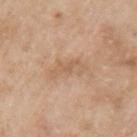Findings:
• follow-up: no biopsy performed (imaged during a skin exam)
• patient: female, aged 73–77
• location: the left upper arm
• tile lighting: white-light illumination
• image: 15 mm crop, total-body photography